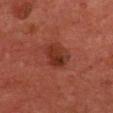Recorded during total-body skin imaging; not selected for excision or biopsy. From the back. Cropped from a total-body skin-imaging series; the visible field is about 15 mm. Automated image analysis of the tile measured an area of roughly 7 mm² and two-axis asymmetry of about 0.25. And it measured a lesion-detection confidence of about 100/100. The recorded lesion diameter is about 3 mm. A male patient aged around 65. Imaged with cross-polarized lighting.